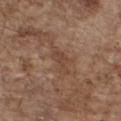Impression: No biopsy was performed on this lesion — it was imaged during a full skin examination and was not determined to be concerning. Context: From the chest. A male subject in their mid-70s. The recorded lesion diameter is about 4 mm. A roughly 15 mm field-of-view crop from a total-body skin photograph. Automated image analysis of the tile measured a lesion color around L≈43 a*≈18 b*≈27 in CIELAB, about 7 CIELAB-L* units darker than the surrounding skin, and a normalized border contrast of about 5.5. And it measured an automated nevus-likeness rating near 0 out of 100 and a lesion-detection confidence of about 90/100.